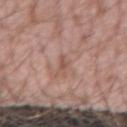Notes:
* follow-up — total-body-photography surveillance lesion; no biopsy
* lighting — white-light
* diameter — about 2.5 mm
* TBP lesion metrics — a lesion area of about 2 mm², an outline eccentricity of about 0.95 (0 = round, 1 = elongated), and two-axis asymmetry of about 0.45; a mean CIELAB color near L≈53 a*≈20 b*≈26, roughly 8 lightness units darker than nearby skin, and a normalized border contrast of about 6; a lesion-detection confidence of about 60/100
* acquisition — 15 mm crop, total-body photography
* location — the arm
* patient — male, roughly 20 years of age